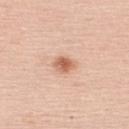follow-up = catalogued during a skin exam; not biopsied
patient = female, aged 38–42
location = the upper back
automated lesion analysis = an area of roughly 4.5 mm², a shape eccentricity near 0.6, and a symmetry-axis asymmetry near 0.2; an average lesion color of about L≈63 a*≈23 b*≈33 (CIELAB), about 13 CIELAB-L* units darker than the surrounding skin, and a normalized border contrast of about 8
tile lighting = white-light illumination
image = 15 mm crop, total-body photography
lesion diameter = about 2.5 mm